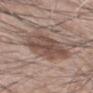<tbp_lesion>
<biopsy_status>not biopsied; imaged during a skin examination</biopsy_status>
<image>
  <source>total-body photography crop</source>
  <field_of_view_mm>15</field_of_view_mm>
</image>
<patient>
  <sex>male</sex>
  <age_approx>60</age_approx>
</patient>
<site>mid back</site>
</tbp_lesion>